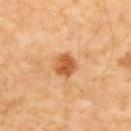Part of a total-body skin-imaging series; this lesion was reviewed on a skin check and was not flagged for biopsy. The lesion is on the back. A male subject, about 55 years old. The tile uses cross-polarized illumination. A 15 mm close-up tile from a total-body photography series done for melanoma screening. The recorded lesion diameter is about 2.5 mm.